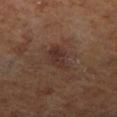Captured during whole-body skin photography for melanoma surveillance; the lesion was not biopsied.
The tile uses cross-polarized illumination.
The lesion is on the right lower leg.
A region of skin cropped from a whole-body photographic capture, roughly 15 mm wide.
A male subject, roughly 70 years of age.